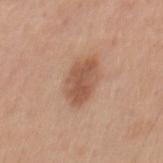Clinical impression: Captured during whole-body skin photography for melanoma surveillance; the lesion was not biopsied. Acquisition and patient details: About 5 mm across. A male patient, aged 28 to 32. The lesion is on the back. A region of skin cropped from a whole-body photographic capture, roughly 15 mm wide.